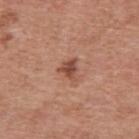{"biopsy_status": "not biopsied; imaged during a skin examination", "patient": {"sex": "male", "age_approx": 55}, "site": "upper back", "lighting": "white-light", "lesion_size": {"long_diameter_mm_approx": 3.0}, "image": {"source": "total-body photography crop", "field_of_view_mm": 15}, "automated_metrics": {"nevus_likeness_0_100": 70, "lesion_detection_confidence_0_100": 100}}